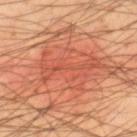follow-up: catalogued during a skin exam; not biopsied | imaging modality: ~15 mm tile from a whole-body skin photo | site: the right lower leg | subject: male, about 45 years old | tile lighting: cross-polarized | lesion size: ~14 mm (longest diameter).